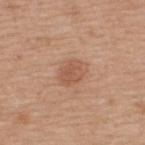The patient is a male in their mid-60s. The lesion is on the upper back. A 15 mm close-up extracted from a 3D total-body photography capture.